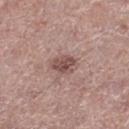biopsy_status: not biopsied; imaged during a skin examination
lesion_size:
  long_diameter_mm_approx: 3.0
site: right lower leg
lighting: white-light
image:
  source: total-body photography crop
  field_of_view_mm: 15
patient:
  sex: male
  age_approx: 55